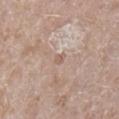{
  "automated_metrics": {
    "border_irregularity_0_10": 2.5,
    "peripheral_color_asymmetry": 0.0,
    "nevus_likeness_0_100": 0
  },
  "site": "right lower leg",
  "patient": {
    "sex": "male",
    "age_approx": 45
  },
  "lighting": "white-light",
  "lesion_size": {
    "long_diameter_mm_approx": 1.0
  },
  "image": {
    "source": "total-body photography crop",
    "field_of_view_mm": 15
  },
  "diagnosis": {
    "histopathology": "seborrheic keratosis",
    "malignancy": "benign",
    "taxonomic_path": [
      "Benign",
      "Benign epidermal proliferations",
      "Seborrheic keratosis"
    ]
  }
}